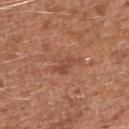The lesion was photographed on a routine skin check and not biopsied; there is no pathology result.
This is a white-light tile.
The subject is a male aged 43–47.
Measured at roughly 3.5 mm in maximum diameter.
On the right upper arm.
This image is a 15 mm lesion crop taken from a total-body photograph.